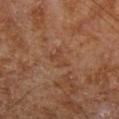This lesion was catalogued during total-body skin photography and was not selected for biopsy. Located on the left lower leg. A 15 mm close-up tile from a total-body photography series done for melanoma screening. The subject is a male aged 68–72.